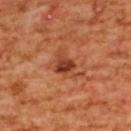Case summary:
* follow-up · no biopsy performed (imaged during a skin exam)
* location · the upper back
* diameter · ≈4 mm
* illumination · cross-polarized
* subject · female, in their mid-50s
* image source · 15 mm crop, total-body photography
* automated lesion analysis · an automated nevus-likeness rating near 40 out of 100 and lesion-presence confidence of about 100/100